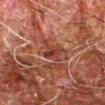Clinical impression: This lesion was catalogued during total-body skin photography and was not selected for biopsy. Image and clinical context: A region of skin cropped from a whole-body photographic capture, roughly 15 mm wide. The patient is a male roughly 80 years of age. On the right forearm.